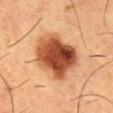Imaged during a routine full-body skin examination; the lesion was not biopsied and no histopathology is available.